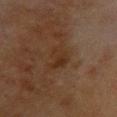No biopsy was performed on this lesion — it was imaged during a full skin examination and was not determined to be concerning.
Imaged with cross-polarized lighting.
A female patient, approximately 80 years of age.
A region of skin cropped from a whole-body photographic capture, roughly 15 mm wide.
The lesion is on the back.